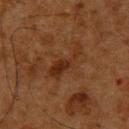The lesion-visualizer software estimated a lesion area of about 7 mm², an eccentricity of roughly 0.95, and a symmetry-axis asymmetry near 0.5. The software also gave about 6 CIELAB-L* units darker than the surrounding skin. It also reported a border-irregularity index near 7/10 and internal color variation of about 2 on a 0–10 scale. It also reported a classifier nevus-likeness of about 5/100 and a lesion-detection confidence of about 100/100. About 5 mm across. From the upper back. This is a cross-polarized tile. Cropped from a total-body skin-imaging series; the visible field is about 15 mm. A male patient, aged 58–62.